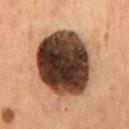{
  "biopsy_status": "not biopsied; imaged during a skin examination",
  "patient": {
    "sex": "male",
    "age_approx": 55
  },
  "site": "chest",
  "lesion_size": {
    "long_diameter_mm_approx": 8.5
  },
  "image": {
    "source": "total-body photography crop",
    "field_of_view_mm": 15
  },
  "automated_metrics": {
    "area_mm2_approx": 45.0,
    "eccentricity": 0.6,
    "shape_asymmetry": 0.1,
    "vs_skin_darker_L": 28.0,
    "vs_skin_contrast_norm": 20.5,
    "color_variation_0_10": 9.0,
    "peripheral_color_asymmetry": 2.5
  }
}